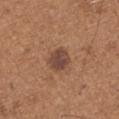Part of a total-body skin-imaging series; this lesion was reviewed on a skin check and was not flagged for biopsy. A male patient roughly 65 years of age. The lesion is located on the left upper arm. The lesion-visualizer software estimated a mean CIELAB color near L≈45 a*≈20 b*≈27, about 11 CIELAB-L* units darker than the surrounding skin, and a lesion-to-skin contrast of about 9 (normalized; higher = more distinct). And it measured radial color variation of about 1. The lesion's longest dimension is about 3 mm. The tile uses white-light illumination. Cropped from a total-body skin-imaging series; the visible field is about 15 mm.